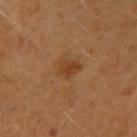The lesion was tiled from a total-body skin photograph and was not biopsied.
Longest diameter approximately 3 mm.
The subject is a male aged 38–42.
A 15 mm crop from a total-body photograph taken for skin-cancer surveillance.
The total-body-photography lesion software estimated an eccentricity of roughly 0.65 and a symmetry-axis asymmetry near 0.25. It also reported a mean CIELAB color near L≈41 a*≈22 b*≈37. And it measured an automated nevus-likeness rating near 50 out of 100 and lesion-presence confidence of about 100/100.
The tile uses cross-polarized illumination.
The lesion is on the right upper arm.